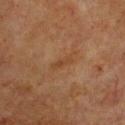| key | value |
|---|---|
| biopsy status | imaged on a skin check; not biopsied |
| site | the chest |
| lighting | cross-polarized illumination |
| acquisition | total-body-photography crop, ~15 mm field of view |
| subject | female, approximately 60 years of age |